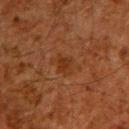Notes:
• follow-up · total-body-photography surveillance lesion; no biopsy
• lesion diameter · about 2.5 mm
• patient · male, about 60 years old
• automated metrics · an area of roughly 4 mm², an eccentricity of roughly 0.7, and a symmetry-axis asymmetry near 0.3; an average lesion color of about L≈25 a*≈20 b*≈28 (CIELAB), a lesion–skin lightness drop of about 5, and a lesion-to-skin contrast of about 6 (normalized; higher = more distinct)
• imaging modality · 15 mm crop, total-body photography
• location · the upper back